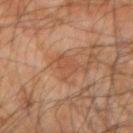| feature | finding |
|---|---|
| workup | catalogued during a skin exam; not biopsied |
| subject | male, roughly 65 years of age |
| lighting | cross-polarized |
| body site | the left forearm |
| image | 15 mm crop, total-body photography |
| TBP lesion metrics | a footprint of about 5.5 mm², a shape eccentricity near 0.5, and a symmetry-axis asymmetry near 0.35; an average lesion color of about L≈43 a*≈20 b*≈29 (CIELAB), about 5 CIELAB-L* units darker than the surrounding skin, and a normalized border contrast of about 4.5; a border-irregularity rating of about 3.5/10, a within-lesion color-variation index near 1.5/10, and peripheral color asymmetry of about 0.5; a classifier nevus-likeness of about 0/100 |
| size | about 3 mm |